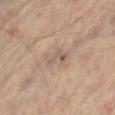Assessment:
This lesion was catalogued during total-body skin photography and was not selected for biopsy.
Image and clinical context:
Automated tile analysis of the lesion measured an area of roughly 4 mm², an eccentricity of roughly 0.85, and two-axis asymmetry of about 0.45. The software also gave border irregularity of about 5 on a 0–10 scale and radial color variation of about 0.5. The software also gave a detector confidence of about 80 out of 100 that the crop contains a lesion. The tile uses cross-polarized illumination. The lesion is on the right thigh. A male patient aged around 70. This image is a 15 mm lesion crop taken from a total-body photograph.